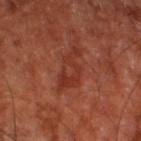image — 15 mm crop, total-body photography
site — the right thigh
patient — roughly 65 years of age
lesion size — ≈5 mm
image-analysis metrics — an area of roughly 8.5 mm², a shape eccentricity near 0.9, and a shape-asymmetry score of about 0.45 (0 = symmetric); a mean CIELAB color near L≈35 a*≈29 b*≈31 and a normalized lesion–skin contrast near 5.5; a border-irregularity index near 6/10, a within-lesion color-variation index near 3.5/10, and peripheral color asymmetry of about 0.5; an automated nevus-likeness rating near 0 out of 100 and a detector confidence of about 100 out of 100 that the crop contains a lesion